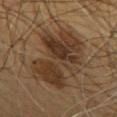Recorded during total-body skin imaging; not selected for excision or biopsy. The recorded lesion diameter is about 8.5 mm. The tile uses cross-polarized illumination. A close-up tile cropped from a whole-body skin photograph, about 15 mm across. The lesion-visualizer software estimated an area of roughly 34 mm², an outline eccentricity of about 0.8 (0 = round, 1 = elongated), and a shape-asymmetry score of about 0.35 (0 = symmetric). And it measured a mean CIELAB color near L≈32 a*≈14 b*≈26, roughly 9 lightness units darker than nearby skin, and a lesion-to-skin contrast of about 9 (normalized; higher = more distinct). A male subject in their mid- to late 50s. On the chest.